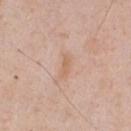Q: Where on the body is the lesion?
A: the front of the torso
Q: Automated lesion metrics?
A: a lesion color around L≈63 a*≈19 b*≈32 in CIELAB and roughly 7 lightness units darker than nearby skin; a color-variation rating of about 0/10 and a peripheral color-asymmetry measure near 0; an automated nevus-likeness rating near 0 out of 100
Q: Illumination type?
A: white-light illumination
Q: What is the imaging modality?
A: 15 mm crop, total-body photography
Q: Lesion size?
A: ~3 mm (longest diameter)
Q: Patient demographics?
A: male, aged 58 to 62